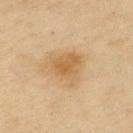The patient is a female aged approximately 55. The lesion's longest dimension is about 3.5 mm. This is a cross-polarized tile. A region of skin cropped from a whole-body photographic capture, roughly 15 mm wide. Located on the upper back.